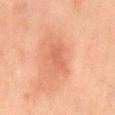  biopsy_status: not biopsied; imaged during a skin examination
  site: back
  lesion_size:
    long_diameter_mm_approx: 3.5
  patient:
    sex: male
    age_approx: 60
  image:
    source: total-body photography crop
    field_of_view_mm: 15
  automated_metrics:
    vs_skin_contrast_norm: 4.5
    nevus_likeness_0_100: 10
    lesion_detection_confidence_0_100: 100
  lighting: cross-polarized